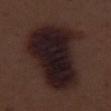biopsy status=total-body-photography surveillance lesion; no biopsy
subject=male, aged 68 to 72
site=the right thigh
TBP lesion metrics=an area of roughly 55 mm² and a symmetry-axis asymmetry near 0.25; an average lesion color of about L≈18 a*≈14 b*≈13 (CIELAB); border irregularity of about 3 on a 0–10 scale, internal color variation of about 6.5 on a 0–10 scale, and a peripheral color-asymmetry measure near 2
illumination=white-light
acquisition=~15 mm crop, total-body skin-cancer survey
lesion size=≈10 mm